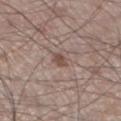A region of skin cropped from a whole-body photographic capture, roughly 15 mm wide. The lesion is on the leg. The lesion-visualizer software estimated a footprint of about 2.5 mm², an outline eccentricity of about 0.85 (0 = round, 1 = elongated), and two-axis asymmetry of about 0.2. And it measured an average lesion color of about L≈48 a*≈17 b*≈24 (CIELAB) and roughly 10 lightness units darker than nearby skin. And it measured a border-irregularity rating of about 2/10 and a color-variation rating of about 1/10. It also reported an automated nevus-likeness rating near 20 out of 100 and a detector confidence of about 100 out of 100 that the crop contains a lesion. A male patient roughly 60 years of age. Captured under white-light illumination.